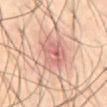Assessment:
Captured during whole-body skin photography for melanoma surveillance; the lesion was not biopsied.
Image and clinical context:
A close-up tile cropped from a whole-body skin photograph, about 15 mm across. The recorded lesion diameter is about 5.5 mm. The lesion is on the abdomen. The tile uses cross-polarized illumination. The total-body-photography lesion software estimated a footprint of about 14 mm² and an eccentricity of roughly 0.7. The software also gave an average lesion color of about L≈60 a*≈23 b*≈25 (CIELAB) and a normalized lesion–skin contrast near 6. The software also gave an automated nevus-likeness rating near 0 out of 100 and lesion-presence confidence of about 100/100. A male patient aged approximately 55.